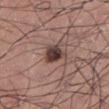<record>
<site>leg</site>
<image>
  <source>total-body photography crop</source>
  <field_of_view_mm>15</field_of_view_mm>
</image>
<lesion_size>
  <long_diameter_mm_approx>3.5</long_diameter_mm_approx>
</lesion_size>
<lighting>white-light</lighting>
<patient>
  <sex>male</sex>
  <age_approx>25</age_approx>
</patient>
<automated_metrics>
  <cielab_L>38</cielab_L>
  <cielab_a>18</cielab_a>
  <cielab_b>20</cielab_b>
  <vs_skin_darker_L>16.0</vs_skin_darker_L>
  <vs_skin_contrast_norm>12.5</vs_skin_contrast_norm>
  <border_irregularity_0_10>3.0</border_irregularity_0_10>
  <color_variation_0_10>5.0</color_variation_0_10>
  <peripheral_color_asymmetry>1.5</peripheral_color_asymmetry>
  <nevus_likeness_0_100>85</nevus_likeness_0_100>
  <lesion_detection_confidence_0_100>100</lesion_detection_confidence_0_100>
</automated_metrics>
</record>